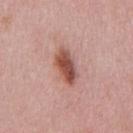biopsy_status: not biopsied; imaged during a skin examination
lesion_size:
  long_diameter_mm_approx: 4.5
lighting: white-light
site: front of the torso
patient:
  sex: male
  age_approx: 50
automated_metrics:
  cielab_L: 52
  cielab_a: 25
  cielab_b: 26
  vs_skin_darker_L: 14.0
  border_irregularity_0_10: 2.0
  color_variation_0_10: 5.5
  peripheral_color_asymmetry: 1.5
  nevus_likeness_0_100: 95
  lesion_detection_confidence_0_100: 100
image:
  source: total-body photography crop
  field_of_view_mm: 15